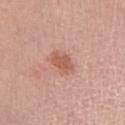notes=total-body-photography surveillance lesion; no biopsy | subject=female, aged 48–52 | image=~15 mm tile from a whole-body skin photo | anatomic site=the chest.